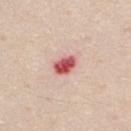| feature | finding |
|---|---|
| follow-up | catalogued during a skin exam; not biopsied |
| image | total-body-photography crop, ~15 mm field of view |
| patient | male, aged around 35 |
| anatomic site | the chest |